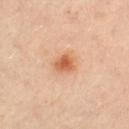Findings:
• follow-up — total-body-photography surveillance lesion; no biopsy
• location — the leg
• image source — ~15 mm tile from a whole-body skin photo
• patient — female, aged 38–42
• tile lighting — cross-polarized